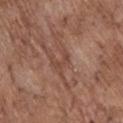biopsy_status: not biopsied; imaged during a skin examination
image:
  source: total-body photography crop
  field_of_view_mm: 15
site: upper back
patient:
  sex: male
  age_approx: 70